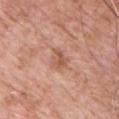Imaged during a routine full-body skin examination; the lesion was not biopsied and no histopathology is available.
The subject is a male aged 58 to 62.
Located on the front of the torso.
A close-up tile cropped from a whole-body skin photograph, about 15 mm across.
The total-body-photography lesion software estimated a footprint of about 3.5 mm² and a shape-asymmetry score of about 0.35 (0 = symmetric). The analysis additionally found roughly 9 lightness units darker than nearby skin and a lesion-to-skin contrast of about 6.5 (normalized; higher = more distinct). The software also gave border irregularity of about 3.5 on a 0–10 scale, a within-lesion color-variation index near 1.5/10, and a peripheral color-asymmetry measure near 0.5.
The tile uses white-light illumination.